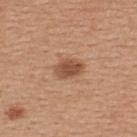Captured during whole-body skin photography for melanoma surveillance; the lesion was not biopsied. Captured under white-light illumination. The lesion is located on the upper back. A female subject, approximately 45 years of age. The lesion's longest dimension is about 3.5 mm. A 15 mm close-up tile from a total-body photography series done for melanoma screening.